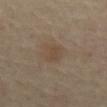* biopsy status · imaged on a skin check; not biopsied
* anatomic site · the abdomen
* imaging modality · total-body-photography crop, ~15 mm field of view
* image-analysis metrics · an outline eccentricity of about 0.65 (0 = round, 1 = elongated) and a shape-asymmetry score of about 0.2 (0 = symmetric); a detector confidence of about 100 out of 100 that the crop contains a lesion
* lighting · cross-polarized
* subject · female, in their 70s
* diameter · about 2.5 mm